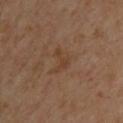Part of a total-body skin-imaging series; this lesion was reviewed on a skin check and was not flagged for biopsy. On the upper back. Cropped from a whole-body photographic skin survey; the tile spans about 15 mm. A male subject in their 50s.